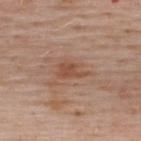Impression:
The lesion was tiled from a total-body skin photograph and was not biopsied.
Acquisition and patient details:
A 15 mm close-up tile from a total-body photography series done for melanoma screening. From the upper back. The subject is a male aged approximately 40. The lesion-visualizer software estimated a border-irregularity rating of about 5/10, a within-lesion color-variation index near 1.5/10, and peripheral color asymmetry of about 0.5.